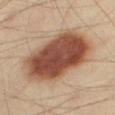Q: Was this lesion biopsied?
A: catalogued during a skin exam; not biopsied
Q: Patient demographics?
A: male, aged 38–42
Q: Where on the body is the lesion?
A: the right thigh
Q: What is the imaging modality?
A: total-body-photography crop, ~15 mm field of view